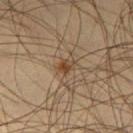Part of a total-body skin-imaging series; this lesion was reviewed on a skin check and was not flagged for biopsy.
A 15 mm close-up tile from a total-body photography series done for melanoma screening.
A male subject roughly 45 years of age.
About 2 mm across.
The lesion-visualizer software estimated a lesion area of about 2.5 mm², an eccentricity of roughly 0.6, and a shape-asymmetry score of about 0.4 (0 = symmetric). And it measured roughly 9 lightness units darker than nearby skin and a normalized border contrast of about 8. The analysis additionally found border irregularity of about 3 on a 0–10 scale, a color-variation rating of about 1.5/10, and a peripheral color-asymmetry measure near 0.5. The analysis additionally found an automated nevus-likeness rating near 85 out of 100 and a lesion-detection confidence of about 100/100.
The lesion is on the left thigh.